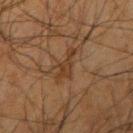Q: Is there a histopathology result?
A: catalogued during a skin exam; not biopsied
Q: What is the anatomic site?
A: the arm
Q: How was the tile lit?
A: cross-polarized illumination
Q: Patient demographics?
A: male, roughly 65 years of age
Q: How large is the lesion?
A: ≈4 mm
Q: What is the imaging modality?
A: ~15 mm tile from a whole-body skin photo